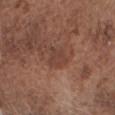biopsy status — no biopsy performed (imaged during a skin exam)
anatomic site — the head or neck
image source — 15 mm crop, total-body photography
size — ~3 mm (longest diameter)
patient — male, aged around 75
illumination — white-light illumination